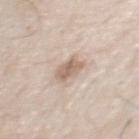Imaged during a routine full-body skin examination; the lesion was not biopsied and no histopathology is available.
The subject is a male about 55 years old.
A lesion tile, about 15 mm wide, cut from a 3D total-body photograph.
On the chest.